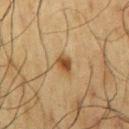Recorded during total-body skin imaging; not selected for excision or biopsy. Located on the right upper arm. A male patient about 60 years old. A 15 mm close-up tile from a total-body photography series done for melanoma screening.